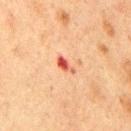{"biopsy_status": "not biopsied; imaged during a skin examination", "image": {"source": "total-body photography crop", "field_of_view_mm": 15}, "patient": {"sex": "male", "age_approx": 75}, "automated_metrics": {"nevus_likeness_0_100": 0, "lesion_detection_confidence_0_100": 100}, "lighting": "cross-polarized", "site": "chest"}